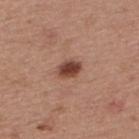Case summary:
- acquisition: ~15 mm tile from a whole-body skin photo
- diameter: ~3 mm (longest diameter)
- image-analysis metrics: a lesion area of about 5 mm² and a shape-asymmetry score of about 0.15 (0 = symmetric); a border-irregularity rating of about 1/10; an automated nevus-likeness rating near 95 out of 100 and lesion-presence confidence of about 100/100
- lighting: white-light illumination
- patient: male, about 55 years old
- location: the back